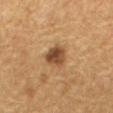workup — no biopsy performed (imaged during a skin exam)
illumination — cross-polarized
imaging modality — total-body-photography crop, ~15 mm field of view
patient — male, aged approximately 65
location — the mid back
TBP lesion metrics — a shape eccentricity near 0.45; a lesion color around L≈38 a*≈17 b*≈29 in CIELAB, roughly 11 lightness units darker than nearby skin, and a lesion-to-skin contrast of about 9.5 (normalized; higher = more distinct); an automated nevus-likeness rating near 85 out of 100 and a detector confidence of about 100 out of 100 that the crop contains a lesion